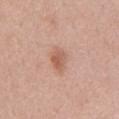The lesion was tiled from a total-body skin photograph and was not biopsied.
A male patient aged around 55.
A 15 mm close-up extracted from a 3D total-body photography capture.